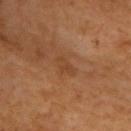Impression:
Captured during whole-body skin photography for melanoma surveillance; the lesion was not biopsied.
Background:
From the upper back. The subject is a male aged 63–67. Cropped from a total-body skin-imaging series; the visible field is about 15 mm. Captured under cross-polarized illumination. Automated image analysis of the tile measured an area of roughly 3.5 mm², a shape eccentricity near 0.8, and two-axis asymmetry of about 0.25. The software also gave a mean CIELAB color near L≈41 a*≈21 b*≈33, roughly 5 lightness units darker than nearby skin, and a normalized lesion–skin contrast near 4.5. The software also gave a classifier nevus-likeness of about 0/100 and a detector confidence of about 100 out of 100 that the crop contains a lesion. Longest diameter approximately 2.5 mm.